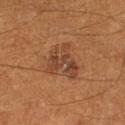Impression:
The lesion was tiled from a total-body skin photograph and was not biopsied.
Image and clinical context:
Measured at roughly 5 mm in maximum diameter. Imaged with cross-polarized lighting. A male subject, aged around 60. The lesion is on the right lower leg. This image is a 15 mm lesion crop taken from a total-body photograph. Automated image analysis of the tile measured a footprint of about 12 mm², an eccentricity of roughly 0.65, and a shape-asymmetry score of about 0.4 (0 = symmetric). The software also gave a border-irregularity rating of about 4.5/10, a color-variation rating of about 5/10, and radial color variation of about 1.5.